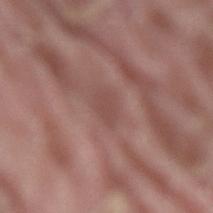biopsy status: no biopsy performed (imaged during a skin exam)
illumination: white-light illumination
acquisition: 15 mm crop, total-body photography
anatomic site: the lower back
diameter: ~3 mm (longest diameter)
subject: male, roughly 40 years of age